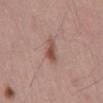| key | value |
|---|---|
| image-analysis metrics | a shape eccentricity near 0.9 and a shape-asymmetry score of about 0.25 (0 = symmetric); a border-irregularity index near 3.5/10 and a peripheral color-asymmetry measure near 1.5 |
| subject | male, in their mid-50s |
| illumination | white-light |
| lesion size | about 4 mm |
| acquisition | ~15 mm crop, total-body skin-cancer survey |